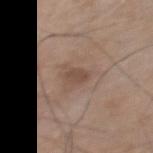Clinical impression:
Imaged during a routine full-body skin examination; the lesion was not biopsied and no histopathology is available.
Context:
A close-up tile cropped from a whole-body skin photograph, about 15 mm across. The total-body-photography lesion software estimated an area of roughly 3 mm², an eccentricity of roughly 0.85, and a shape-asymmetry score of about 0.25 (0 = symmetric). The analysis additionally found a border-irregularity index near 2.5/10, a within-lesion color-variation index near 1/10, and peripheral color asymmetry of about 0.5. The software also gave an automated nevus-likeness rating near 0 out of 100 and a detector confidence of about 100 out of 100 that the crop contains a lesion. The patient is a male aged approximately 75. Located on the mid back.